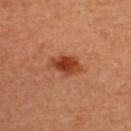Q: Was this lesion biopsied?
A: imaged on a skin check; not biopsied
Q: Lesion location?
A: the upper back
Q: What kind of image is this?
A: ~15 mm crop, total-body skin-cancer survey
Q: What is the lesion's diameter?
A: about 4 mm
Q: What did automated image analysis measure?
A: an area of roughly 7.5 mm² and two-axis asymmetry of about 0.2; a border-irregularity index near 2/10, a within-lesion color-variation index near 4.5/10, and peripheral color asymmetry of about 1.5; a nevus-likeness score of about 100/100 and a lesion-detection confidence of about 100/100
Q: Patient demographics?
A: female, roughly 30 years of age